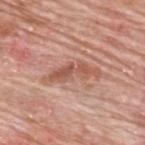follow-up=catalogued during a skin exam; not biopsied | location=the upper back | image=total-body-photography crop, ~15 mm field of view | tile lighting=white-light | size=about 6 mm | patient=male, in their 80s.